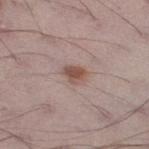Clinical impression: Recorded during total-body skin imaging; not selected for excision or biopsy. Acquisition and patient details: Automated image analysis of the tile measured an average lesion color of about L≈51 a*≈18 b*≈24 (CIELAB) and a lesion–skin lightness drop of about 11. The analysis additionally found a within-lesion color-variation index near 3/10 and a peripheral color-asymmetry measure near 1. The subject is a male aged 68 to 72. Cropped from a total-body skin-imaging series; the visible field is about 15 mm. On the right thigh. The tile uses white-light illumination.